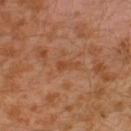Part of a total-body skin-imaging series; this lesion was reviewed on a skin check and was not flagged for biopsy. Cropped from a total-body skin-imaging series; the visible field is about 15 mm. The recorded lesion diameter is about 2.5 mm. A male patient roughly 30 years of age. Captured under cross-polarized illumination. The lesion is located on the left leg. An algorithmic analysis of the crop reported a lesion area of about 2 mm², an outline eccentricity of about 0.95 (0 = round, 1 = elongated), and a symmetry-axis asymmetry near 0.4. It also reported a lesion color around L≈44 a*≈24 b*≈35 in CIELAB and a normalized lesion–skin contrast near 6. The software also gave an automated nevus-likeness rating near 0 out of 100 and a detector confidence of about 100 out of 100 that the crop contains a lesion.